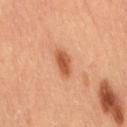Imaged during a routine full-body skin examination; the lesion was not biopsied and no histopathology is available. Located on the right thigh. A female patient, roughly 60 years of age. Cropped from a total-body skin-imaging series; the visible field is about 15 mm.